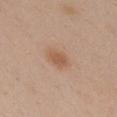<record>
<biopsy_status>not biopsied; imaged during a skin examination</biopsy_status>
<site>chest</site>
<image>
  <source>total-body photography crop</source>
  <field_of_view_mm>15</field_of_view_mm>
</image>
<patient>
  <sex>female</sex>
  <age_approx>25</age_approx>
</patient>
</record>